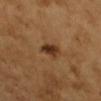follow-up: no biopsy performed (imaged during a skin exam)
anatomic site: the right upper arm
lighting: cross-polarized illumination
subject: female, aged 68–72
TBP lesion metrics: an area of roughly 4.5 mm² and an outline eccentricity of about 0.8 (0 = round, 1 = elongated); a lesion color around L≈36 a*≈20 b*≈33 in CIELAB and a lesion-to-skin contrast of about 10.5 (normalized; higher = more distinct); lesion-presence confidence of about 100/100
imaging modality: 15 mm crop, total-body photography